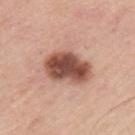Q: Was a biopsy performed?
A: catalogued during a skin exam; not biopsied
Q: Lesion location?
A: the upper back
Q: Who is the patient?
A: male, aged 43–47
Q: How was this image acquired?
A: 15 mm crop, total-body photography